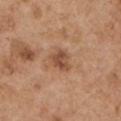Recorded during total-body skin imaging; not selected for excision or biopsy.
A male subject, about 55 years old.
This is a white-light tile.
Measured at roughly 2.5 mm in maximum diameter.
The lesion is on the left upper arm.
Cropped from a total-body skin-imaging series; the visible field is about 15 mm.